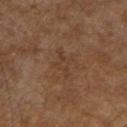No biopsy was performed on this lesion — it was imaged during a full skin examination and was not determined to be concerning. A 15 mm crop from a total-body photograph taken for skin-cancer surveillance. The subject is a male aged 83 or over. The recorded lesion diameter is about 3.5 mm. The tile uses cross-polarized illumination. The lesion is located on the right forearm.